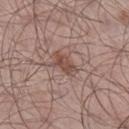<lesion>
<biopsy_status>not biopsied; imaged during a skin examination</biopsy_status>
<lighting>white-light</lighting>
<site>right lower leg</site>
<automated_metrics>
  <area_mm2_approx>5.5</area_mm2_approx>
  <eccentricity>0.85</eccentricity>
  <shape_asymmetry>0.3</shape_asymmetry>
  <border_irregularity_0_10>3.5</border_irregularity_0_10>
  <nevus_likeness_0_100>50</nevus_likeness_0_100>
  <lesion_detection_confidence_0_100>100</lesion_detection_confidence_0_100>
</automated_metrics>
<image>
  <source>total-body photography crop</source>
  <field_of_view_mm>15</field_of_view_mm>
</image>
<patient>
  <sex>male</sex>
  <age_approx>55</age_approx>
</patient>
<lesion_size>
  <long_diameter_mm_approx>3.5</long_diameter_mm_approx>
</lesion_size>
</lesion>